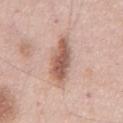– tile lighting: white-light
– size: about 7.5 mm
– subject: male, about 55 years old
– site: the chest
– image source: ~15 mm crop, total-body skin-cancer survey